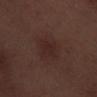Part of a total-body skin-imaging series; this lesion was reviewed on a skin check and was not flagged for biopsy.
A 15 mm crop from a total-body photograph taken for skin-cancer surveillance.
About 3 mm across.
Located on the right lower leg.
A male subject aged around 70.